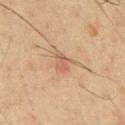notes — imaged on a skin check; not biopsied
size — ~3 mm (longest diameter)
illumination — cross-polarized illumination
acquisition — ~15 mm crop, total-body skin-cancer survey
patient — male, aged 58 to 62